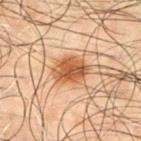Assessment:
This lesion was catalogued during total-body skin photography and was not selected for biopsy.
Context:
A male subject, aged approximately 50. A region of skin cropped from a whole-body photographic capture, roughly 15 mm wide. This is a cross-polarized tile. The lesion's longest dimension is about 3.5 mm. An algorithmic analysis of the crop reported an area of roughly 8.5 mm² and a shape-asymmetry score of about 0.15 (0 = symmetric). The analysis additionally found border irregularity of about 1.5 on a 0–10 scale, a within-lesion color-variation index near 4/10, and radial color variation of about 1.5. It also reported lesion-presence confidence of about 100/100. On the chest.